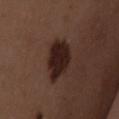Assessment:
No biopsy was performed on this lesion — it was imaged during a full skin examination and was not determined to be concerning.
Clinical summary:
Measured at roughly 5 mm in maximum diameter. The patient is a female aged approximately 50. Imaged with white-light lighting. The lesion is located on the chest. A 15 mm close-up extracted from a 3D total-body photography capture.